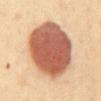Assessment: The lesion was photographed on a routine skin check and not biopsied; there is no pathology result. Context: Captured under cross-polarized illumination. About 8.5 mm across. Located on the abdomen. The subject is a female in their 40s. An algorithmic analysis of the crop reported a footprint of about 44 mm². The analysis additionally found a mean CIELAB color near L≈48 a*≈21 b*≈28. It also reported border irregularity of about 1 on a 0–10 scale and a color-variation rating of about 5/10. A region of skin cropped from a whole-body photographic capture, roughly 15 mm wide.